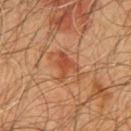Assessment: Captured during whole-body skin photography for melanoma surveillance; the lesion was not biopsied. Background: A 15 mm close-up tile from a total-body photography series done for melanoma screening. The lesion's longest dimension is about 3.5 mm. A male patient, roughly 60 years of age. Located on the chest.